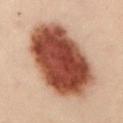| feature | finding |
|---|---|
| imaging modality | 15 mm crop, total-body photography |
| subject | female, approximately 30 years of age |
| anatomic site | the abdomen |
| size | ~10.5 mm (longest diameter) |